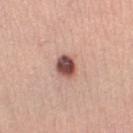Notes:
• notes: no biopsy performed (imaged during a skin exam)
• size: ~3 mm (longest diameter)
• image source: total-body-photography crop, ~15 mm field of view
• patient: female, in their mid- to late 40s
• TBP lesion metrics: a mean CIELAB color near L≈50 a*≈23 b*≈24, a lesion–skin lightness drop of about 19, and a normalized border contrast of about 12.5; a classifier nevus-likeness of about 80/100 and a lesion-detection confidence of about 100/100
• anatomic site: the right lower leg
• illumination: white-light